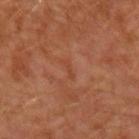anatomic site = the left forearm
subject = male, in their 30s
acquisition = 15 mm crop, total-body photography
lesion diameter = ≈2 mm